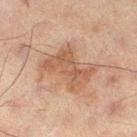This lesion was catalogued during total-body skin photography and was not selected for biopsy. From the left leg. Captured under cross-polarized illumination. A male subject aged 58–62. Approximately 6 mm at its widest. This image is a 15 mm lesion crop taken from a total-body photograph.